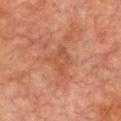Impression: Recorded during total-body skin imaging; not selected for excision or biopsy. Context: A male patient, aged around 75. About 3.5 mm across. A lesion tile, about 15 mm wide, cut from a 3D total-body photograph. Located on the chest.